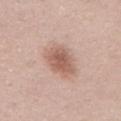The lesion was photographed on a routine skin check and not biopsied; there is no pathology result. Located on the abdomen. A region of skin cropped from a whole-body photographic capture, roughly 15 mm wide. A male subject roughly 55 years of age. Imaged with white-light lighting. The lesion's longest dimension is about 5 mm.